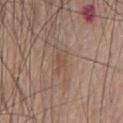The lesion was tiled from a total-body skin photograph and was not biopsied.
The subject is a male aged approximately 80.
About 3 mm across.
The lesion is located on the chest.
The tile uses white-light illumination.
The lesion-visualizer software estimated a footprint of about 4 mm², an eccentricity of roughly 0.85, and two-axis asymmetry of about 0.4.
A 15 mm close-up extracted from a 3D total-body photography capture.